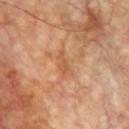Q: Was a biopsy performed?
A: imaged on a skin check; not biopsied
Q: What are the patient's age and sex?
A: male, aged 58 to 62
Q: Lesion size?
A: ≈3 mm
Q: What is the imaging modality?
A: 15 mm crop, total-body photography
Q: How was the tile lit?
A: cross-polarized
Q: Where on the body is the lesion?
A: the chest